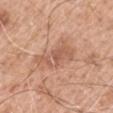notes = no biopsy performed (imaged during a skin exam)
patient = male, about 60 years old
site = the right upper arm
image = ~15 mm crop, total-body skin-cancer survey
lesion diameter = ≈5.5 mm
lighting = white-light